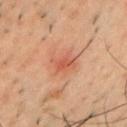{
  "biopsy_status": "not biopsied; imaged during a skin examination",
  "patient": {
    "sex": "male",
    "age_approx": 50
  },
  "site": "front of the torso",
  "image": {
    "source": "total-body photography crop",
    "field_of_view_mm": 15
  }
}